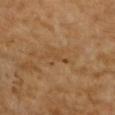Q: Where on the body is the lesion?
A: the right upper arm
Q: What is the imaging modality?
A: ~15 mm tile from a whole-body skin photo
Q: How large is the lesion?
A: about 3.5 mm
Q: Patient demographics?
A: female, aged approximately 70